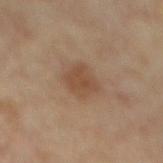No biopsy was performed on this lesion — it was imaged during a full skin examination and was not determined to be concerning.
Approximately 3 mm at its widest.
The subject is a male aged around 85 or older.
On the mid back.
Captured under cross-polarized illumination.
A 15 mm close-up tile from a total-body photography series done for melanoma screening.